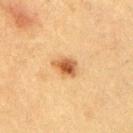biopsy_status: not biopsied; imaged during a skin examination
lighting: cross-polarized
image:
  source: total-body photography crop
  field_of_view_mm: 15
lesion_size:
  long_diameter_mm_approx: 3.0
site: right upper arm
patient:
  sex: male
  age_approx: 50